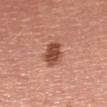Imaged during a routine full-body skin examination; the lesion was not biopsied and no histopathology is available. The patient is a female roughly 50 years of age. This is a white-light tile. A lesion tile, about 15 mm wide, cut from a 3D total-body photograph. The lesion is on the left thigh. Measured at roughly 4 mm in maximum diameter. The total-body-photography lesion software estimated a lesion area of about 7.5 mm² and a symmetry-axis asymmetry near 0.2. The analysis additionally found an automated nevus-likeness rating near 65 out of 100 and a detector confidence of about 100 out of 100 that the crop contains a lesion.